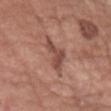Captured during whole-body skin photography for melanoma surveillance; the lesion was not biopsied. The tile uses white-light illumination. A close-up tile cropped from a whole-body skin photograph, about 15 mm across. A female subject, in their 80s. The lesion is on the head or neck. Measured at roughly 4.5 mm in maximum diameter.